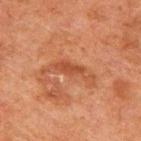Context:
The patient is a male aged 58–62. The total-body-photography lesion software estimated a lesion-detection confidence of about 100/100. A region of skin cropped from a whole-body photographic capture, roughly 15 mm wide. About 6 mm across. Located on the upper back.